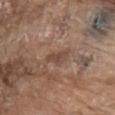biopsy_status: not biopsied; imaged during a skin examination
lesion_size:
  long_diameter_mm_approx: 3.0
site: front of the torso
image:
  source: total-body photography crop
  field_of_view_mm: 15
patient:
  sex: male
  age_approx: 80